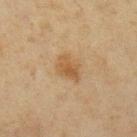Clinical impression: Captured during whole-body skin photography for melanoma surveillance; the lesion was not biopsied.